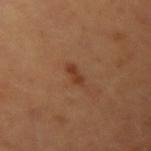Q: Was this lesion biopsied?
A: total-body-photography surveillance lesion; no biopsy
Q: What is the anatomic site?
A: the left arm
Q: What is the imaging modality?
A: total-body-photography crop, ~15 mm field of view
Q: What lighting was used for the tile?
A: cross-polarized illumination
Q: What is the lesion's diameter?
A: about 2.5 mm
Q: Patient demographics?
A: male, aged approximately 50